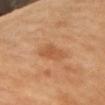Captured during whole-body skin photography for melanoma surveillance; the lesion was not biopsied. Located on the left arm. This is a cross-polarized tile. The subject is a female aged 58 to 62. About 3.5 mm across. Cropped from a total-body skin-imaging series; the visible field is about 15 mm.